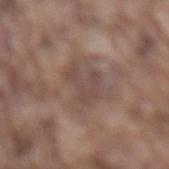Captured during whole-body skin photography for melanoma surveillance; the lesion was not biopsied. This image is a 15 mm lesion crop taken from a total-body photograph. Automated tile analysis of the lesion measured an area of roughly 8.5 mm² and two-axis asymmetry of about 0.45. The patient is a male in their mid-70s. Located on the lower back. This is a white-light tile. Measured at roughly 5 mm in maximum diameter.